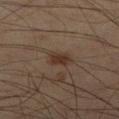Impression: This lesion was catalogued during total-body skin photography and was not selected for biopsy. Image and clinical context: The total-body-photography lesion software estimated an average lesion color of about L≈28 a*≈15 b*≈22 (CIELAB) and a lesion-to-skin contrast of about 8.5 (normalized; higher = more distinct). It also reported border irregularity of about 3 on a 0–10 scale and radial color variation of about 0.5. A male subject, approximately 60 years of age. A 15 mm close-up tile from a total-body photography series done for melanoma screening. On the leg. About 3 mm across.